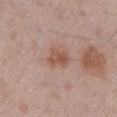biopsy_status: not biopsied; imaged during a skin examination
site: left lower leg
image:
  source: total-body photography crop
  field_of_view_mm: 15
patient:
  sex: male
  age_approx: 60